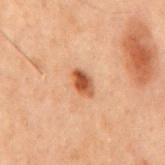Context:
The lesion's longest dimension is about 3 mm. A 15 mm crop from a total-body photograph taken for skin-cancer surveillance. A male patient roughly 70 years of age. The lesion is located on the mid back. Imaged with cross-polarized lighting. The lesion-visualizer software estimated roughly 12 lightness units darker than nearby skin.